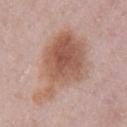The lesion is on the left upper arm. This is a white-light tile. About 10 mm across. Cropped from a whole-body photographic skin survey; the tile spans about 15 mm. A female patient roughly 30 years of age.